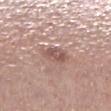Findings:
- workup: imaged on a skin check; not biopsied
- subject: male, approximately 55 years of age
- acquisition: ~15 mm tile from a whole-body skin photo
- image-analysis metrics: a lesion area of about 6 mm², an eccentricity of roughly 0.55, and two-axis asymmetry of about 0.25; a lesion–skin lightness drop of about 10 and a normalized lesion–skin contrast near 6.5; a border-irregularity rating of about 2/10, a color-variation rating of about 3.5/10, and radial color variation of about 1; a nevus-likeness score of about 0/100
- body site: the right lower leg
- lesion size: about 3 mm
- lighting: white-light illumination